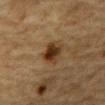Recorded during total-body skin imaging; not selected for excision or biopsy. A close-up tile cropped from a whole-body skin photograph, about 15 mm across. The patient is a male in their mid-80s or older. On the front of the torso. The lesion-visualizer software estimated a shape eccentricity near 0.5. The software also gave a lesion color around L≈30 a*≈16 b*≈28 in CIELAB and a lesion–skin lightness drop of about 11. The software also gave a border-irregularity index near 2/10, a color-variation rating of about 5.5/10, and radial color variation of about 2. Approximately 3 mm at its widest.